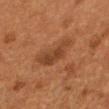This lesion was catalogued during total-body skin photography and was not selected for biopsy. Located on the head or neck. A male subject in their 60s. Longest diameter approximately 5 mm. Cropped from a whole-body photographic skin survey; the tile spans about 15 mm. The tile uses cross-polarized illumination. The lesion-visualizer software estimated an average lesion color of about L≈32 a*≈18 b*≈27 (CIELAB), a lesion–skin lightness drop of about 7, and a normalized border contrast of about 7.